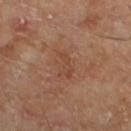Q: Was this lesion biopsied?
A: total-body-photography surveillance lesion; no biopsy
Q: How large is the lesion?
A: ≈3 mm
Q: Automated lesion metrics?
A: a lesion color around L≈45 a*≈21 b*≈31 in CIELAB and about 6 CIELAB-L* units darker than the surrounding skin
Q: Who is the patient?
A: male, aged 63 to 67
Q: What kind of image is this?
A: ~15 mm tile from a whole-body skin photo
Q: Where on the body is the lesion?
A: the left lower leg
Q: What lighting was used for the tile?
A: cross-polarized